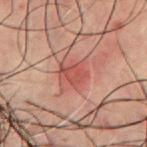• follow-up · imaged on a skin check; not biopsied
• location · the chest
• size · about 3 mm
• automated lesion analysis · a border-irregularity index near 2/10, a within-lesion color-variation index near 2.5/10, and radial color variation of about 1
• acquisition · ~15 mm tile from a whole-body skin photo
• patient · male, roughly 60 years of age
• tile lighting · cross-polarized